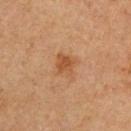Notes:
– follow-up · catalogued during a skin exam; not biopsied
– acquisition · 15 mm crop, total-body photography
– patient · male, aged 63–67
– site · the left upper arm